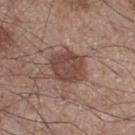Assessment: The lesion was tiled from a total-body skin photograph and was not biopsied. Clinical summary: From the right thigh. Cropped from a whole-body photographic skin survey; the tile spans about 15 mm. A male subject aged around 60. The total-body-photography lesion software estimated an area of roughly 13 mm², an outline eccentricity of about 0.45 (0 = round, 1 = elongated), and a symmetry-axis asymmetry near 0.15. And it measured an average lesion color of about L≈45 a*≈19 b*≈24 (CIELAB), a lesion–skin lightness drop of about 11, and a lesion-to-skin contrast of about 8.5 (normalized; higher = more distinct). The analysis additionally found a border-irregularity rating of about 2/10, a color-variation rating of about 2.5/10, and peripheral color asymmetry of about 1. And it measured a classifier nevus-likeness of about 45/100 and lesion-presence confidence of about 100/100.